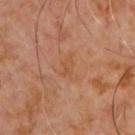biopsy status: catalogued during a skin exam; not biopsied | imaging modality: 15 mm crop, total-body photography | body site: the chest | patient: male, aged around 60.